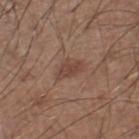Captured during whole-body skin photography for melanoma surveillance; the lesion was not biopsied.
A 15 mm close-up tile from a total-body photography series done for melanoma screening.
Captured under white-light illumination.
The lesion-visualizer software estimated a shape eccentricity near 0.85 and a shape-asymmetry score of about 0.35 (0 = symmetric). And it measured an average lesion color of about L≈43 a*≈19 b*≈25 (CIELAB), about 8 CIELAB-L* units darker than the surrounding skin, and a normalized border contrast of about 6.5. The analysis additionally found a border-irregularity rating of about 3.5/10, a color-variation rating of about 2/10, and a peripheral color-asymmetry measure near 0.5.
On the leg.
The lesion's longest dimension is about 3 mm.
A male patient roughly 60 years of age.